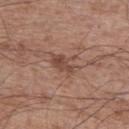Clinical impression:
No biopsy was performed on this lesion — it was imaged during a full skin examination and was not determined to be concerning.
Acquisition and patient details:
A male patient, approximately 65 years of age. A 15 mm crop from a total-body photograph taken for skin-cancer surveillance. On the left upper arm.